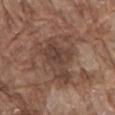biopsy status=total-body-photography surveillance lesion; no biopsy
site=the front of the torso
lighting=white-light
subject=male, about 80 years old
imaging modality=~15 mm crop, total-body skin-cancer survey
lesion diameter=≈7.5 mm